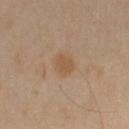| key | value |
|---|---|
| biopsy status | no biopsy performed (imaged during a skin exam) |
| patient | male, aged 48 to 52 |
| image | ~15 mm crop, total-body skin-cancer survey |
| location | the left upper arm |
| tile lighting | cross-polarized illumination |
| automated metrics | an eccentricity of roughly 0.55; a normalized border contrast of about 6; a classifier nevus-likeness of about 20/100 and lesion-presence confidence of about 100/100 |